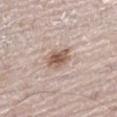Captured during whole-body skin photography for melanoma surveillance; the lesion was not biopsied.
A 15 mm close-up extracted from a 3D total-body photography capture.
A male patient aged 68 to 72.
On the right leg.